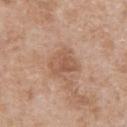follow-up = catalogued during a skin exam; not biopsied
lesion diameter = ~3.5 mm (longest diameter)
subject = male, in their 50s
location = the chest
tile lighting = white-light illumination
acquisition = ~15 mm tile from a whole-body skin photo